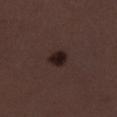Q: Is there a histopathology result?
A: no biopsy performed (imaged during a skin exam)
Q: What lighting was used for the tile?
A: white-light
Q: What is the imaging modality?
A: ~15 mm crop, total-body skin-cancer survey
Q: Lesion location?
A: the leg
Q: What did automated image analysis measure?
A: a mean CIELAB color near L≈19 a*≈14 b*≈15 and roughly 10 lightness units darker than nearby skin; a classifier nevus-likeness of about 80/100 and a detector confidence of about 100 out of 100 that the crop contains a lesion
Q: How large is the lesion?
A: ≈2.5 mm
Q: Patient demographics?
A: female, aged 48–52